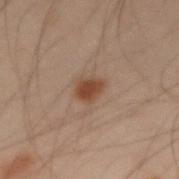{"biopsy_status": "not biopsied; imaged during a skin examination", "automated_metrics": {"cielab_L": 35, "cielab_a": 17, "cielab_b": 25, "vs_skin_darker_L": 9.0, "lesion_detection_confidence_0_100": 100}, "image": {"source": "total-body photography crop", "field_of_view_mm": 15}, "patient": {"sex": "male", "age_approx": 50}, "site": "left arm"}